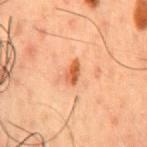The lesion was tiled from a total-body skin photograph and was not biopsied.
Approximately 3 mm at its widest.
This is a cross-polarized tile.
From the front of the torso.
Automated image analysis of the tile measured a border-irregularity index near 1.5/10 and a within-lesion color-variation index near 3/10.
A male subject aged 48–52.
A region of skin cropped from a whole-body photographic capture, roughly 15 mm wide.